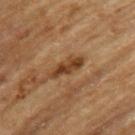Part of a total-body skin-imaging series; this lesion was reviewed on a skin check and was not flagged for biopsy. This is a cross-polarized tile. A male subject, approximately 65 years of age. On the upper back. The lesion's longest dimension is about 4.5 mm. Cropped from a whole-body photographic skin survey; the tile spans about 15 mm.